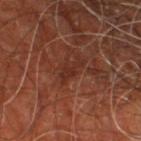<case>
<biopsy_status>not biopsied; imaged during a skin examination</biopsy_status>
<image>
  <source>total-body photography crop</source>
  <field_of_view_mm>15</field_of_view_mm>
</image>
<patient>
  <sex>male</sex>
  <age_approx>60</age_approx>
</patient>
<site>leg</site>
</case>